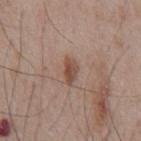notes=no biopsy performed (imaged during a skin exam); imaging modality=~15 mm tile from a whole-body skin photo; body site=the chest; patient=male, about 55 years old.